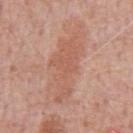biopsy_status: not biopsied; imaged during a skin examination
image:
  source: total-body photography crop
  field_of_view_mm: 15
site: chest
patient:
  sex: male
  age_approx: 60